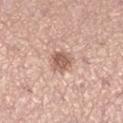The lesion was tiled from a total-body skin photograph and was not biopsied.
Approximately 3 mm at its widest.
A roughly 15 mm field-of-view crop from a total-body skin photograph.
On the left lower leg.
A female subject approximately 35 years of age.
Imaged with white-light lighting.
Automated tile analysis of the lesion measured a lesion color around L≈60 a*≈21 b*≈27 in CIELAB, roughly 12 lightness units darker than nearby skin, and a lesion-to-skin contrast of about 7.5 (normalized; higher = more distinct). It also reported border irregularity of about 2 on a 0–10 scale and radial color variation of about 1.5. The analysis additionally found a nevus-likeness score of about 80/100.